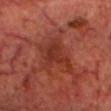| key | value |
|---|---|
| biopsy status | imaged on a skin check; not biopsied |
| automated lesion analysis | a footprint of about 11 mm², an eccentricity of roughly 0.65, and a shape-asymmetry score of about 0.5 (0 = symmetric); a lesion–skin lightness drop of about 7 and a normalized border contrast of about 6.5 |
| imaging modality | ~15 mm crop, total-body skin-cancer survey |
| lesion size | about 5 mm |
| tile lighting | cross-polarized illumination |
| anatomic site | the head or neck |
| subject | male, in their 70s |